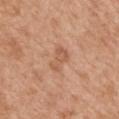Q: Was this lesion biopsied?
A: catalogued during a skin exam; not biopsied
Q: Lesion size?
A: ~3 mm (longest diameter)
Q: What lighting was used for the tile?
A: white-light
Q: How was this image acquired?
A: 15 mm crop, total-body photography
Q: What are the patient's age and sex?
A: female, roughly 40 years of age
Q: What is the anatomic site?
A: the mid back